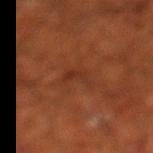This lesion was catalogued during total-body skin photography and was not selected for biopsy. On the left lower leg. Cropped from a whole-body photographic skin survey; the tile spans about 15 mm. The tile uses cross-polarized illumination. Approximately 3.5 mm at its widest. The total-body-photography lesion software estimated an eccentricity of roughly 0.95 and two-axis asymmetry of about 0.5. The analysis additionally found an automated nevus-likeness rating near 0 out of 100 and a lesion-detection confidence of about 95/100. The subject is a male roughly 70 years of age.